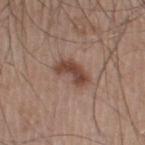Clinical impression:
Imaged during a routine full-body skin examination; the lesion was not biopsied and no histopathology is available.
Clinical summary:
On the right thigh. This is a white-light tile. Automated tile analysis of the lesion measured a lesion area of about 7.5 mm², an eccentricity of roughly 0.8, and a shape-asymmetry score of about 0.35 (0 = symmetric). It also reported an average lesion color of about L≈45 a*≈19 b*≈26 (CIELAB), about 11 CIELAB-L* units darker than the surrounding skin, and a normalized lesion–skin contrast near 8.5. The analysis additionally found a within-lesion color-variation index near 3/10 and a peripheral color-asymmetry measure near 1. About 4 mm across. The patient is a male about 45 years old. A 15 mm crop from a total-body photograph taken for skin-cancer surveillance.